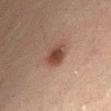follow-up = no biopsy performed (imaged during a skin exam)